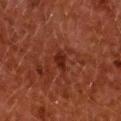Clinical impression:
Recorded during total-body skin imaging; not selected for excision or biopsy.
Clinical summary:
The total-body-photography lesion software estimated an area of roughly 3 mm², a shape eccentricity near 0.75, and a shape-asymmetry score of about 0.3 (0 = symmetric). And it measured a lesion color around L≈22 a*≈24 b*≈25 in CIELAB, a lesion–skin lightness drop of about 7, and a normalized lesion–skin contrast near 7.5. The software also gave a border-irregularity rating of about 3/10, internal color variation of about 1 on a 0–10 scale, and radial color variation of about 0.5. And it measured a classifier nevus-likeness of about 25/100 and a lesion-detection confidence of about 100/100. Approximately 2.5 mm at its widest. Cropped from a total-body skin-imaging series; the visible field is about 15 mm. A female patient, aged 48 to 52. The lesion is on the upper back. Imaged with cross-polarized lighting.